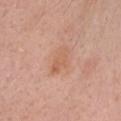Assessment:
Captured during whole-body skin photography for melanoma surveillance; the lesion was not biopsied.
Background:
This is a white-light tile. A male subject aged 48–52. About 3 mm across. The lesion is located on the head or neck. An algorithmic analysis of the crop reported an eccentricity of roughly 0.65 and a shape-asymmetry score of about 0.3 (0 = symmetric). The analysis additionally found border irregularity of about 3 on a 0–10 scale and a within-lesion color-variation index near 3/10. And it measured a nevus-likeness score of about 5/100 and a lesion-detection confidence of about 100/100. This image is a 15 mm lesion crop taken from a total-body photograph.